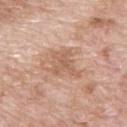Clinical impression: The lesion was tiled from a total-body skin photograph and was not biopsied. Background: A 15 mm close-up extracted from a 3D total-body photography capture. Imaged with white-light lighting. On the back. A male patient aged 58–62. The recorded lesion diameter is about 4.5 mm.